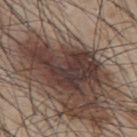Assessment:
Captured during whole-body skin photography for melanoma surveillance; the lesion was not biopsied.
Clinical summary:
A 15 mm close-up extracted from a 3D total-body photography capture. Automated tile analysis of the lesion measured a footprint of about 24 mm², a shape eccentricity near 0.85, and a symmetry-axis asymmetry near 0.5. The analysis additionally found an automated nevus-likeness rating near 50 out of 100 and lesion-presence confidence of about 20/100. A male patient in their mid- to late 40s. The lesion is on the upper back. This is a white-light tile.